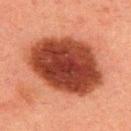location = the upper back | automated lesion analysis = a border-irregularity rating of about 1.5/10, a within-lesion color-variation index near 5/10, and a peripheral color-asymmetry measure near 1.5 | tile lighting = cross-polarized | image = total-body-photography crop, ~15 mm field of view | patient = male, about 50 years old.